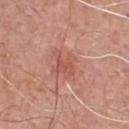size = ~3.5 mm (longest diameter)
automated metrics = a footprint of about 6.5 mm² and a symmetry-axis asymmetry near 0.3; roughly 8 lightness units darker than nearby skin; a nevus-likeness score of about 30/100 and a lesion-detection confidence of about 100/100
imaging modality = ~15 mm tile from a whole-body skin photo
subject = male, aged approximately 50
location = the chest